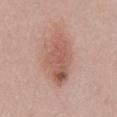Impression:
The lesion was tiled from a total-body skin photograph and was not biopsied.
Image and clinical context:
Longest diameter approximately 7 mm. A roughly 15 mm field-of-view crop from a total-body skin photograph. This is a white-light tile. A male patient in their mid-50s. Located on the front of the torso. The lesion-visualizer software estimated a border-irregularity rating of about 2.5/10, a within-lesion color-variation index near 6.5/10, and radial color variation of about 2.5. It also reported an automated nevus-likeness rating near 90 out of 100 and a lesion-detection confidence of about 100/100.